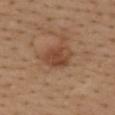follow-up: imaged on a skin check; not biopsied | lesion size: ~4 mm (longest diameter) | location: the upper back | subject: female, aged 38 to 42 | illumination: white-light illumination | acquisition: total-body-photography crop, ~15 mm field of view.